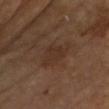Imaged with cross-polarized lighting. Automated tile analysis of the lesion measured an area of roughly 9 mm² and two-axis asymmetry of about 0.35. And it measured an average lesion color of about L≈31 a*≈16 b*≈25 (CIELAB), a lesion–skin lightness drop of about 5, and a lesion-to-skin contrast of about 5 (normalized; higher = more distinct). The software also gave a border-irregularity index near 4/10, internal color variation of about 1.5 on a 0–10 scale, and peripheral color asymmetry of about 0.5. The analysis additionally found a classifier nevus-likeness of about 0/100 and a lesion-detection confidence of about 100/100. A roughly 15 mm field-of-view crop from a total-body skin photograph. About 5 mm across. On the chest. A female subject, roughly 65 years of age.